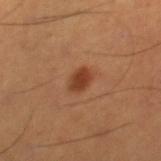  biopsy_status: not biopsied; imaged during a skin examination
  image:
    source: total-body photography crop
    field_of_view_mm: 15
  patient:
    sex: male
    age_approx: 50
  site: right thigh
  lighting: cross-polarized
  lesion_size:
    long_diameter_mm_approx: 2.5
  automated_metrics:
    border_irregularity_0_10: 2.0
    color_variation_0_10: 2.0
    lesion_detection_confidence_0_100: 100